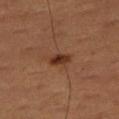No biopsy was performed on this lesion — it was imaged during a full skin examination and was not determined to be concerning. Cropped from a whole-body photographic skin survey; the tile spans about 15 mm. A male subject, roughly 50 years of age. Located on the right thigh.